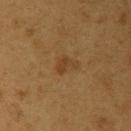Q: Was a biopsy performed?
A: no biopsy performed (imaged during a skin exam)
Q: How was this image acquired?
A: total-body-photography crop, ~15 mm field of view
Q: What did automated image analysis measure?
A: a mean CIELAB color near L≈35 a*≈16 b*≈32 and a normalized border contrast of about 6; a classifier nevus-likeness of about 10/100 and lesion-presence confidence of about 100/100
Q: Lesion location?
A: the right upper arm
Q: What is the lesion's diameter?
A: ~3 mm (longest diameter)
Q: What lighting was used for the tile?
A: cross-polarized illumination
Q: What are the patient's age and sex?
A: male, about 55 years old